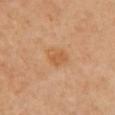Q: Is there a histopathology result?
A: imaged on a skin check; not biopsied
Q: What lighting was used for the tile?
A: cross-polarized illumination
Q: What are the patient's age and sex?
A: male, approximately 65 years of age
Q: What is the anatomic site?
A: the chest
Q: How was this image acquired?
A: ~15 mm tile from a whole-body skin photo
Q: How large is the lesion?
A: ~3 mm (longest diameter)
Q: Automated lesion metrics?
A: an area of roughly 5.5 mm² and a shape eccentricity near 0.7